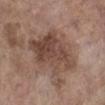<tbp_lesion>
<biopsy_status>not biopsied; imaged during a skin examination</biopsy_status>
<lesion_size>
  <long_diameter_mm_approx>6.5</long_diameter_mm_approx>
</lesion_size>
<automated_metrics>
  <vs_skin_darker_L>10.0</vs_skin_darker_L>
</automated_metrics>
<site>left lower leg</site>
<image>
  <source>total-body photography crop</source>
  <field_of_view_mm>15</field_of_view_mm>
</image>
<lighting>white-light</lighting>
<patient>
  <sex>female</sex>
  <age_approx>85+</age_approx>
</patient>
</tbp_lesion>